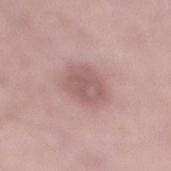The lesion was photographed on a routine skin check and not biopsied; there is no pathology result.
This image is a 15 mm lesion crop taken from a total-body photograph.
The tile uses white-light illumination.
An algorithmic analysis of the crop reported roughly 10 lightness units darker than nearby skin. It also reported border irregularity of about 1.5 on a 0–10 scale, a color-variation rating of about 2.5/10, and a peripheral color-asymmetry measure near 1. The software also gave a nevus-likeness score of about 20/100.
Approximately 4 mm at its widest.
A male patient, aged 48–52.
Located on the leg.